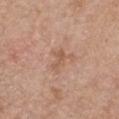{
  "biopsy_status": "not biopsied; imaged during a skin examination",
  "lighting": "white-light",
  "site": "chest",
  "patient": {
    "sex": "female",
    "age_approx": 40
  },
  "image": {
    "source": "total-body photography crop",
    "field_of_view_mm": 15
  },
  "automated_metrics": {
    "cielab_L": 56,
    "cielab_a": 21,
    "cielab_b": 31,
    "vs_skin_darker_L": 7.0,
    "vs_skin_contrast_norm": 5.5,
    "color_variation_0_10": 0.5,
    "peripheral_color_asymmetry": 0.0,
    "nevus_likeness_0_100": 0
  }
}